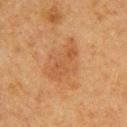Notes:
- notes — catalogued during a skin exam; not biopsied
- subject — female, aged around 60
- image — ~15 mm crop, total-body skin-cancer survey
- site — the back
- tile lighting — cross-polarized illumination
- diameter — ~6 mm (longest diameter)